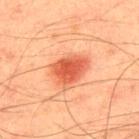Captured during whole-body skin photography for melanoma surveillance; the lesion was not biopsied. The total-body-photography lesion software estimated a border-irregularity index near 2.5/10 and peripheral color asymmetry of about 0.5. It also reported a classifier nevus-likeness of about 100/100. A male subject aged around 45. This image is a 15 mm lesion crop taken from a total-body photograph. Approximately 4.5 mm at its widest. From the upper back.